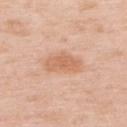| feature | finding |
|---|---|
| biopsy status | no biopsy performed (imaged during a skin exam) |
| subject | female, about 50 years old |
| diameter | ~4 mm (longest diameter) |
| acquisition | total-body-photography crop, ~15 mm field of view |
| tile lighting | white-light |
| anatomic site | the upper back |